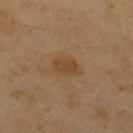A region of skin cropped from a whole-body photographic capture, roughly 15 mm wide. The subject is a male aged 43–47. The lesion is on the upper back.